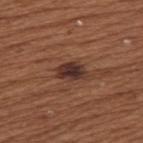{"biopsy_status": "not biopsied; imaged during a skin examination", "lesion_size": {"long_diameter_mm_approx": 3.5}, "lighting": "white-light", "patient": {"sex": "female", "age_approx": 65}, "image": {"source": "total-body photography crop", "field_of_view_mm": 15}, "site": "back"}